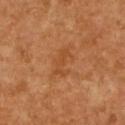  biopsy_status: not biopsied; imaged during a skin examination
  automated_metrics:
    cielab_L: 48
    cielab_a: 27
    cielab_b: 41
  patient:
    sex: female
    age_approx: 55
  lesion_size:
    long_diameter_mm_approx: 3.5
  image:
    source: total-body photography crop
    field_of_view_mm: 15
  site: chest
  lighting: cross-polarized